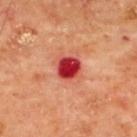* subject · male, in their mid- to late 60s
* diameter · ≈2.5 mm
* automated metrics · a footprint of about 6 mm², an outline eccentricity of about 0.3 (0 = round, 1 = elongated), and a shape-asymmetry score of about 0.15 (0 = symmetric); a normalized lesion–skin contrast near 13.5; a classifier nevus-likeness of about 0/100 and a lesion-detection confidence of about 100/100
* location · the upper back
* tile lighting · cross-polarized
* imaging modality · 15 mm crop, total-body photography